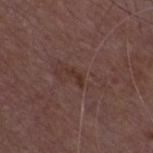Imaged during a routine full-body skin examination; the lesion was not biopsied and no histopathology is available.